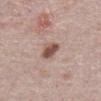Q: Where on the body is the lesion?
A: the abdomen
Q: Patient demographics?
A: male, aged approximately 75
Q: How was this image acquired?
A: ~15 mm tile from a whole-body skin photo